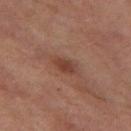The lesion was photographed on a routine skin check and not biopsied; there is no pathology result. Automated image analysis of the tile measured about 8 CIELAB-L* units darker than the surrounding skin and a lesion-to-skin contrast of about 7 (normalized; higher = more distinct). And it measured a border-irregularity rating of about 2/10, a within-lesion color-variation index near 2.5/10, and a peripheral color-asymmetry measure near 1. And it measured a nevus-likeness score of about 40/100 and a detector confidence of about 100 out of 100 that the crop contains a lesion. The subject is a female roughly 60 years of age. Approximately 3 mm at its widest. A region of skin cropped from a whole-body photographic capture, roughly 15 mm wide. Captured under cross-polarized illumination. The lesion is located on the leg.